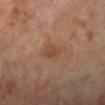Q: Patient demographics?
A: male, aged approximately 70
Q: What is the anatomic site?
A: the left lower leg
Q: What is the imaging modality?
A: 15 mm crop, total-body photography